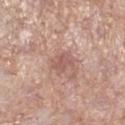| field | value |
|---|---|
| site | the right lower leg |
| patient | female, about 70 years old |
| image | 15 mm crop, total-body photography |